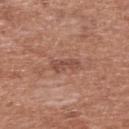<lesion>
<biopsy_status>not biopsied; imaged during a skin examination</biopsy_status>
<image>
  <source>total-body photography crop</source>
  <field_of_view_mm>15</field_of_view_mm>
</image>
<lighting>white-light</lighting>
<automated_metrics>
  <area_mm2_approx>4.0</area_mm2_approx>
  <shape_asymmetry>0.35</shape_asymmetry>
  <cielab_L>48</cielab_L>
  <cielab_a>23</cielab_a>
  <cielab_b>27</cielab_b>
  <vs_skin_darker_L>8.0</vs_skin_darker_L>
  <vs_skin_contrast_norm>6.5</vs_skin_contrast_norm>
  <color_variation_0_10>2.5</color_variation_0_10>
  <peripheral_color_asymmetry>1.0</peripheral_color_asymmetry>
  <nevus_likeness_0_100>0</nevus_likeness_0_100>
  <lesion_detection_confidence_0_100>100</lesion_detection_confidence_0_100>
</automated_metrics>
<site>upper back</site>
<lesion_size>
  <long_diameter_mm_approx>3.0</long_diameter_mm_approx>
</lesion_size>
<patient>
  <sex>male</sex>
  <age_approx>55</age_approx>
</patient>
</lesion>